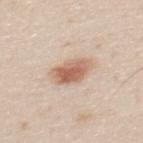Clinical impression: This lesion was catalogued during total-body skin photography and was not selected for biopsy. Background: The lesion's longest dimension is about 4 mm. The lesion is located on the mid back. A male patient, aged around 25. Automated image analysis of the tile measured a lesion area of about 9 mm², an outline eccentricity of about 0.7 (0 = round, 1 = elongated), and two-axis asymmetry of about 0.2. It also reported an automated nevus-likeness rating near 100 out of 100 and a lesion-detection confidence of about 100/100. This image is a 15 mm lesion crop taken from a total-body photograph.